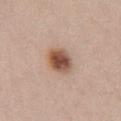Clinical impression:
Imaged during a routine full-body skin examination; the lesion was not biopsied and no histopathology is available.
Acquisition and patient details:
About 3.5 mm across. The total-body-photography lesion software estimated a lesion-to-skin contrast of about 10.5 (normalized; higher = more distinct). And it measured border irregularity of about 1.5 on a 0–10 scale. And it measured a lesion-detection confidence of about 100/100. A 15 mm close-up tile from a total-body photography series done for melanoma screening. The subject is a female aged 38 to 42. On the chest. Imaged with white-light lighting.